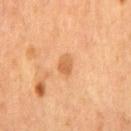location = the chest | subject = male, aged approximately 55 | image = 15 mm crop, total-body photography.